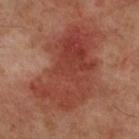Imaged with cross-polarized lighting.
On the right lower leg.
A male patient aged 68 to 72.
Cropped from a whole-body photographic skin survey; the tile spans about 15 mm.